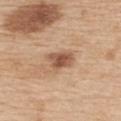The lesion was tiled from a total-body skin photograph and was not biopsied.